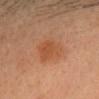Case summary:
* biopsy status — no biopsy performed (imaged during a skin exam)
* imaging modality — ~15 mm crop, total-body skin-cancer survey
* lesion diameter — ≈4 mm
* subject — female, aged 28 to 32
* body site — the head or neck